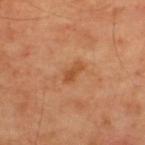| feature | finding |
|---|---|
| biopsy status | total-body-photography surveillance lesion; no biopsy |
| size | ~3 mm (longest diameter) |
| tile lighting | cross-polarized illumination |
| subject | male, approximately 60 years of age |
| body site | the upper back |
| acquisition | total-body-photography crop, ~15 mm field of view |